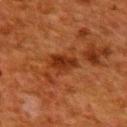No biopsy was performed on this lesion — it was imaged during a full skin examination and was not determined to be concerning. The lesion is located on the upper back. The subject is a female aged approximately 50. Captured under cross-polarized illumination. Measured at roughly 3.5 mm in maximum diameter. This image is a 15 mm lesion crop taken from a total-body photograph. The lesion-visualizer software estimated a footprint of about 6 mm², an outline eccentricity of about 0.8 (0 = round, 1 = elongated), and a shape-asymmetry score of about 0.25 (0 = symmetric). And it measured a mean CIELAB color near L≈28 a*≈25 b*≈32 and a lesion-to-skin contrast of about 8.5 (normalized; higher = more distinct).